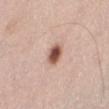Q: Was this lesion biopsied?
A: catalogued during a skin exam; not biopsied
Q: What is the imaging modality?
A: ~15 mm tile from a whole-body skin photo
Q: How large is the lesion?
A: about 2.5 mm
Q: What did automated image analysis measure?
A: a lesion color around L≈54 a*≈22 b*≈27 in CIELAB, about 18 CIELAB-L* units darker than the surrounding skin, and a normalized lesion–skin contrast near 11.5; internal color variation of about 5 on a 0–10 scale and a peripheral color-asymmetry measure near 1.5
Q: Who is the patient?
A: female, aged approximately 65
Q: Where on the body is the lesion?
A: the abdomen